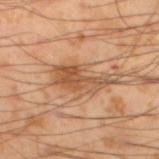* notes — total-body-photography surveillance lesion; no biopsy
* size — about 6.5 mm
* image source — ~15 mm crop, total-body skin-cancer survey
* tile lighting — cross-polarized
* site — the left lower leg
* subject — male, aged approximately 55